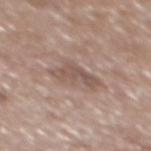The lesion was tiled from a total-body skin photograph and was not biopsied. On the back. Automated image analysis of the tile measured a footprint of about 7 mm², an eccentricity of roughly 0.85, and a symmetry-axis asymmetry near 0.45. The software also gave a detector confidence of about 100 out of 100 that the crop contains a lesion. A male subject approximately 65 years of age. This image is a 15 mm lesion crop taken from a total-body photograph. Measured at roughly 4.5 mm in maximum diameter.